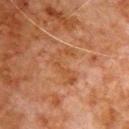Part of a total-body skin-imaging series; this lesion was reviewed on a skin check and was not flagged for biopsy.
Cropped from a whole-body photographic skin survey; the tile spans about 15 mm.
Located on the chest.
Imaged with cross-polarized lighting.
An algorithmic analysis of the crop reported a lesion area of about 6 mm², a shape eccentricity near 0.85, and a shape-asymmetry score of about 0.75 (0 = symmetric). The software also gave a mean CIELAB color near L≈39 a*≈21 b*≈31, about 5 CIELAB-L* units darker than the surrounding skin, and a lesion-to-skin contrast of about 5.5 (normalized; higher = more distinct). And it measured a within-lesion color-variation index near 0/10 and a peripheral color-asymmetry measure near 0. It also reported a nevus-likeness score of about 0/100.
The lesion's longest dimension is about 4.5 mm.
A male patient, aged around 80.